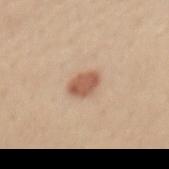Q: Is there a histopathology result?
A: total-body-photography surveillance lesion; no biopsy
Q: Illumination type?
A: white-light
Q: Lesion location?
A: the mid back
Q: Automated lesion metrics?
A: an outline eccentricity of about 0.65 (0 = round, 1 = elongated) and two-axis asymmetry of about 0.15; border irregularity of about 1.5 on a 0–10 scale and a peripheral color-asymmetry measure near 1
Q: What kind of image is this?
A: ~15 mm crop, total-body skin-cancer survey
Q: Patient demographics?
A: female, in their mid- to late 40s
Q: Lesion size?
A: ~3 mm (longest diameter)